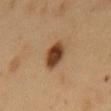follow-up: catalogued during a skin exam; not biopsied
imaging modality: ~15 mm crop, total-body skin-cancer survey
patient: male, approximately 50 years of age
location: the front of the torso